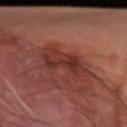  biopsy_status: not biopsied; imaged during a skin examination